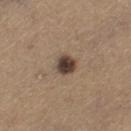notes: catalogued during a skin exam; not biopsied
image-analysis metrics: an average lesion color of about L≈41 a*≈14 b*≈23 (CIELAB), about 16 CIELAB-L* units darker than the surrounding skin, and a lesion-to-skin contrast of about 13 (normalized; higher = more distinct); a border-irregularity rating of about 2/10, a color-variation rating of about 4.5/10, and a peripheral color-asymmetry measure near 1
location: the left thigh
image source: 15 mm crop, total-body photography
lesion size: about 2.5 mm
lighting: white-light
subject: male, aged 63 to 67